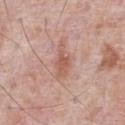Imaged during a routine full-body skin examination; the lesion was not biopsied and no histopathology is available. From the chest. The tile uses white-light illumination. Automated tile analysis of the lesion measured a lesion area of about 5.5 mm², a shape eccentricity near 0.9, and two-axis asymmetry of about 0.4. The software also gave a classifier nevus-likeness of about 20/100 and lesion-presence confidence of about 100/100. A male patient, aged approximately 70. A region of skin cropped from a whole-body photographic capture, roughly 15 mm wide.